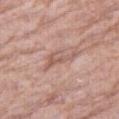Notes:
- notes — catalogued during a skin exam; not biopsied
- location — the right thigh
- TBP lesion metrics — an area of roughly 5.5 mm² and a shape-asymmetry score of about 0.6 (0 = symmetric); a border-irregularity rating of about 7.5/10 and a color-variation rating of about 2/10; an automated nevus-likeness rating near 0 out of 100
- patient — male, aged 78–82
- acquisition — ~15 mm crop, total-body skin-cancer survey
- lighting — white-light illumination